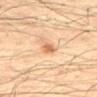Captured during whole-body skin photography for melanoma surveillance; the lesion was not biopsied. Imaged with cross-polarized lighting. On the mid back. A male patient aged 58 to 62. Cropped from a total-body skin-imaging series; the visible field is about 15 mm. The lesion-visualizer software estimated a nevus-likeness score of about 75/100 and a lesion-detection confidence of about 100/100. Longest diameter approximately 2 mm.